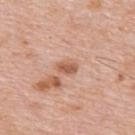Q: Is there a histopathology result?
A: total-body-photography surveillance lesion; no biopsy
Q: What is the anatomic site?
A: the upper back
Q: Who is the patient?
A: male, aged 78 to 82
Q: How large is the lesion?
A: ≈2.5 mm
Q: How was this image acquired?
A: ~15 mm tile from a whole-body skin photo